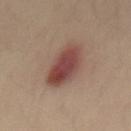This lesion was catalogued during total-body skin photography and was not selected for biopsy. This is a cross-polarized tile. A male patient, in their mid- to late 30s. A 15 mm close-up tile from a total-body photography series done for melanoma screening. The recorded lesion diameter is about 5.5 mm. Located on the mid back.